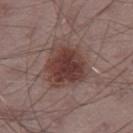follow-up: no biopsy performed (imaged during a skin exam) | diameter: ~5.5 mm (longest diameter) | site: the right thigh | patient: male, in their mid-60s | lighting: white-light | image source: 15 mm crop, total-body photography | automated lesion analysis: a border-irregularity index near 2/10 and a within-lesion color-variation index near 4/10; a nevus-likeness score of about 90/100 and lesion-presence confidence of about 100/100.